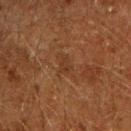<case>
  <biopsy_status>not biopsied; imaged during a skin examination</biopsy_status>
  <site>right upper arm</site>
  <patient>
    <sex>male</sex>
    <age_approx>60</age_approx>
  </patient>
  <lighting>cross-polarized</lighting>
  <image>
    <source>total-body photography crop</source>
    <field_of_view_mm>15</field_of_view_mm>
  </image>
  <automated_metrics>
    <cielab_L>27</cielab_L>
    <cielab_a>17</cielab_a>
    <cielab_b>26</cielab_b>
    <vs_skin_darker_L>4.0</vs_skin_darker_L>
    <vs_skin_contrast_norm>4.5</vs_skin_contrast_norm>
    <nevus_likeness_0_100>0</nevus_likeness_0_100>
    <lesion_detection_confidence_0_100>95</lesion_detection_confidence_0_100>
  </automated_metrics>
  <lesion_size>
    <long_diameter_mm_approx>3.5</long_diameter_mm_approx>
  </lesion_size>
</case>